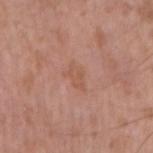Q: Was a biopsy performed?
A: imaged on a skin check; not biopsied
Q: Where on the body is the lesion?
A: the right upper arm
Q: What kind of image is this?
A: 15 mm crop, total-body photography
Q: Patient demographics?
A: male, approximately 50 years of age